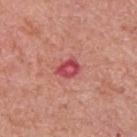<tbp_lesion>
  <patient>
    <sex>male</sex>
    <age_approx>70</age_approx>
  </patient>
  <site>upper back</site>
  <image>
    <source>total-body photography crop</source>
    <field_of_view_mm>15</field_of_view_mm>
  </image>
  <lesion_size>
    <long_diameter_mm_approx>3.0</long_diameter_mm_approx>
  </lesion_size>
  <automated_metrics>
    <nevus_likeness_0_100>0</nevus_likeness_0_100>
    <lesion_detection_confidence_0_100>100</lesion_detection_confidence_0_100>
  </automated_metrics>
  <lighting>white-light</lighting>
</tbp_lesion>